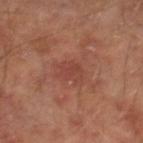The lesion was photographed on a routine skin check and not biopsied; there is no pathology result.
A male patient aged around 65.
From the right lower leg.
The lesion-visualizer software estimated an outline eccentricity of about 0.55 (0 = round, 1 = elongated) and a symmetry-axis asymmetry near 0.25. The analysis additionally found an average lesion color of about L≈42 a*≈26 b*≈26 (CIELAB).
The recorded lesion diameter is about 2.5 mm.
Cropped from a whole-body photographic skin survey; the tile spans about 15 mm.
Imaged with cross-polarized lighting.